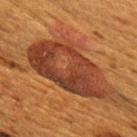Approximately 8.5 mm at its widest. Cropped from a total-body skin-imaging series; the visible field is about 15 mm. The lesion is on the back. The lesion-visualizer software estimated a lesion area of about 37 mm², a shape eccentricity near 0.75, and two-axis asymmetry of about 0.25. It also reported a mean CIELAB color near L≈33 a*≈23 b*≈29, about 11 CIELAB-L* units darker than the surrounding skin, and a normalized border contrast of about 10.5. It also reported a border-irregularity index near 3.5/10, a color-variation rating of about 7/10, and radial color variation of about 2.5. The software also gave an automated nevus-likeness rating near 75 out of 100 and lesion-presence confidence of about 75/100. A female subject, in their 50s.